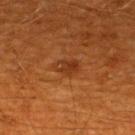* biopsy status — imaged on a skin check; not biopsied